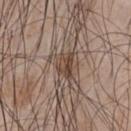Q: Was this lesion biopsied?
A: imaged on a skin check; not biopsied
Q: How large is the lesion?
A: ~3.5 mm (longest diameter)
Q: Illumination type?
A: white-light
Q: Where on the body is the lesion?
A: the chest
Q: How was this image acquired?
A: ~15 mm tile from a whole-body skin photo
Q: What are the patient's age and sex?
A: male, about 45 years old